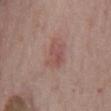Notes:
– biopsy status · imaged on a skin check; not biopsied
– acquisition · 15 mm crop, total-body photography
– tile lighting · white-light illumination
– patient · male, aged around 75
– lesion diameter · ≈3.5 mm
– location · the mid back